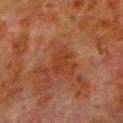biopsy status: catalogued during a skin exam; not biopsied
image: total-body-photography crop, ~15 mm field of view
body site: the left lower leg
TBP lesion metrics: border irregularity of about 3 on a 0–10 scale and peripheral color asymmetry of about 0.5; a nevus-likeness score of about 0/100 and a detector confidence of about 100 out of 100 that the crop contains a lesion
lighting: cross-polarized illumination
subject: male, aged 78 to 82
lesion size: about 3 mm